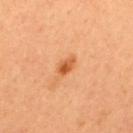Approximately 2.5 mm at its widest.
An algorithmic analysis of the crop reported an area of roughly 3 mm², a shape eccentricity near 0.8, and two-axis asymmetry of about 0.25. It also reported a lesion color around L≈58 a*≈30 b*≈45 in CIELAB, a lesion–skin lightness drop of about 12, and a normalized border contrast of about 8.5. The software also gave internal color variation of about 5.5 on a 0–10 scale and a peripheral color-asymmetry measure near 2.5. And it measured an automated nevus-likeness rating near 95 out of 100 and lesion-presence confidence of about 100/100.
A 15 mm crop from a total-body photograph taken for skin-cancer surveillance.
Imaged with cross-polarized lighting.
The patient is a female roughly 50 years of age.